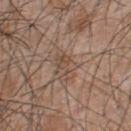biopsy_status: not biopsied; imaged during a skin examination
site: upper back
patient:
  sex: male
  age_approx: 45
image:
  source: total-body photography crop
  field_of_view_mm: 15